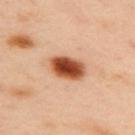Captured during whole-body skin photography for melanoma surveillance; the lesion was not biopsied. The lesion is on the upper back. A lesion tile, about 15 mm wide, cut from a 3D total-body photograph. Longest diameter approximately 4.5 mm. Captured under cross-polarized illumination. The total-body-photography lesion software estimated two-axis asymmetry of about 0.15. It also reported a mean CIELAB color near L≈53 a*≈28 b*≈38, roughly 21 lightness units darker than nearby skin, and a normalized lesion–skin contrast near 13.5. The software also gave a within-lesion color-variation index near 7.5/10 and radial color variation of about 2. A female subject, approximately 40 years of age.